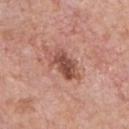The lesion was tiled from a total-body skin photograph and was not biopsied. The lesion is located on the chest. A roughly 15 mm field-of-view crop from a total-body skin photograph. Captured under white-light illumination. A male patient, aged 58–62. The recorded lesion diameter is about 5 mm.